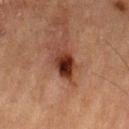Impression:
Captured during whole-body skin photography for melanoma surveillance; the lesion was not biopsied.
Image and clinical context:
The lesion is located on the left thigh. A male patient, about 85 years old or older. Longest diameter approximately 3.5 mm. Imaged with cross-polarized lighting. A 15 mm close-up extracted from a 3D total-body photography capture.